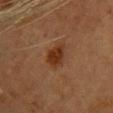Automated image analysis of the tile measured a border-irregularity index near 2.5/10, a within-lesion color-variation index near 2/10, and peripheral color asymmetry of about 0.5. The subject is a female aged 58 to 62. Captured under cross-polarized illumination. A close-up tile cropped from a whole-body skin photograph, about 15 mm across. From the upper back.